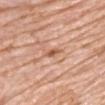No biopsy was performed on this lesion — it was imaged during a full skin examination and was not determined to be concerning. Imaged with white-light lighting. Longest diameter approximately 2.5 mm. The subject is a male approximately 75 years of age. A 15 mm close-up extracted from a 3D total-body photography capture. The total-body-photography lesion software estimated border irregularity of about 4.5 on a 0–10 scale and radial color variation of about 0. It also reported a nevus-likeness score of about 0/100 and lesion-presence confidence of about 100/100. The lesion is on the front of the torso.